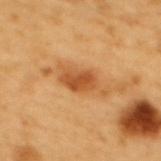Findings:
– workup — imaged on a skin check; not biopsied
– diameter — ~5 mm (longest diameter)
– subject — female, aged around 55
– anatomic site — the upper back
– image source — total-body-photography crop, ~15 mm field of view